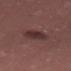Notes:
• workup — no biopsy performed (imaged during a skin exam)
• subject — female, aged around 40
• site — the right thigh
• size — ≈4 mm
• acquisition — total-body-photography crop, ~15 mm field of view
• automated lesion analysis — a footprint of about 8 mm², a shape eccentricity near 0.65, and two-axis asymmetry of about 0.2; a mean CIELAB color near L≈32 a*≈19 b*≈18, roughly 8 lightness units darker than nearby skin, and a lesion-to-skin contrast of about 7.5 (normalized; higher = more distinct)